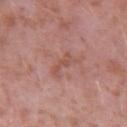Q: Was a biopsy performed?
A: imaged on a skin check; not biopsied
Q: Lesion location?
A: the arm
Q: How was the tile lit?
A: white-light illumination
Q: What is the lesion's diameter?
A: about 4 mm
Q: What is the imaging modality?
A: 15 mm crop, total-body photography
Q: Patient demographics?
A: male, in their 40s
Q: What did automated image analysis measure?
A: roughly 7 lightness units darker than nearby skin; a classifier nevus-likeness of about 0/100 and lesion-presence confidence of about 100/100